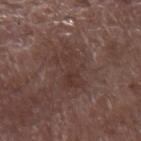biopsy_status: not biopsied; imaged during a skin examination
lighting: white-light
image:
  source: total-body photography crop
  field_of_view_mm: 15
site: arm
patient:
  sex: male
  age_approx: 75
automated_metrics:
  border_irregularity_0_10: 8.5
  color_variation_0_10: 2.5
  peripheral_color_asymmetry: 1.0
  nevus_likeness_0_100: 0